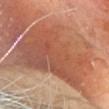The lesion was photographed on a routine skin check and not biopsied; there is no pathology result. A lesion tile, about 15 mm wide, cut from a 3D total-body photograph. The lesion's longest dimension is about 13.5 mm. A female subject in their mid- to late 70s. The tile uses cross-polarized illumination. The lesion is located on the head or neck.